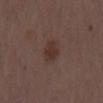| key | value |
|---|---|
| biopsy status | total-body-photography surveillance lesion; no biopsy |
| subject | male, approximately 70 years of age |
| lesion size | ≈3.5 mm |
| lighting | white-light illumination |
| site | the right upper arm |
| imaging modality | total-body-photography crop, ~15 mm field of view |